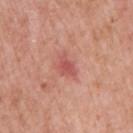follow-up — no biopsy performed (imaged during a skin exam)
anatomic site — the right upper arm
image-analysis metrics — a footprint of about 3.5 mm², an outline eccentricity of about 0.75 (0 = round, 1 = elongated), and a shape-asymmetry score of about 0.35 (0 = symmetric); a color-variation rating of about 1.5/10
subject — male, aged 58 to 62
lighting — white-light
acquisition — total-body-photography crop, ~15 mm field of view
lesion size — ~2.5 mm (longest diameter)